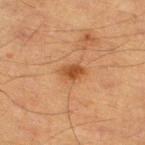Clinical summary:
Approximately 3 mm at its widest. The patient is a male aged around 85 or older. The lesion is on the right thigh. A 15 mm crop from a total-body photograph taken for skin-cancer surveillance. Captured under cross-polarized illumination.